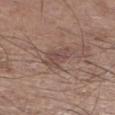Clinical impression: The lesion was photographed on a routine skin check and not biopsied; there is no pathology result. Context: The tile uses white-light illumination. On the right lower leg. A close-up tile cropped from a whole-body skin photograph, about 15 mm across. The recorded lesion diameter is about 3.5 mm. An algorithmic analysis of the crop reported a lesion area of about 7 mm², a shape eccentricity near 0.65, and a symmetry-axis asymmetry near 0.35. It also reported a border-irregularity index near 4/10, a color-variation rating of about 2.5/10, and radial color variation of about 1. A male patient about 60 years old.